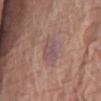Q: Was this lesion biopsied?
A: catalogued during a skin exam; not biopsied
Q: Lesion location?
A: the leg
Q: Automated lesion metrics?
A: a lesion area of about 6 mm²; an automated nevus-likeness rating near 0 out of 100
Q: What kind of image is this?
A: 15 mm crop, total-body photography
Q: How was the tile lit?
A: white-light
Q: What are the patient's age and sex?
A: female, roughly 75 years of age
Q: What is the lesion's diameter?
A: ≈3.5 mm